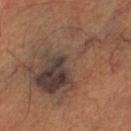Imaged during a routine full-body skin examination; the lesion was not biopsied and no histopathology is available. The patient is a male aged around 60. A region of skin cropped from a whole-body photographic capture, roughly 15 mm wide. From the left lower leg.